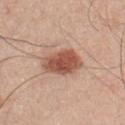| field | value |
|---|---|
| biopsy status | catalogued during a skin exam; not biopsied |
| size | about 5 mm |
| location | the chest |
| automated metrics | an average lesion color of about L≈53 a*≈24 b*≈29 (CIELAB), a lesion–skin lightness drop of about 15, and a lesion-to-skin contrast of about 10 (normalized; higher = more distinct); an automated nevus-likeness rating near 100 out of 100 and a detector confidence of about 100 out of 100 that the crop contains a lesion |
| tile lighting | white-light illumination |
| image | 15 mm crop, total-body photography |
| subject | male, in their mid-40s |